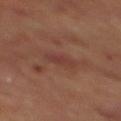{
  "biopsy_status": "not biopsied; imaged during a skin examination",
  "site": "mid back",
  "lesion_size": {
    "long_diameter_mm_approx": 2.5
  },
  "patient": {
    "sex": "male",
    "age_approx": 70
  },
  "lighting": "cross-polarized",
  "image": {
    "source": "total-body photography crop",
    "field_of_view_mm": 15
  }
}